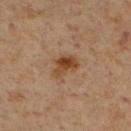biopsy status: total-body-photography surveillance lesion; no biopsy
tile lighting: cross-polarized illumination
patient: male, roughly 60 years of age
automated metrics: an area of roughly 5.5 mm², an outline eccentricity of about 0.8 (0 = round, 1 = elongated), and two-axis asymmetry of about 0.4; a lesion color around L≈41 a*≈19 b*≈32 in CIELAB and roughly 10 lightness units darker than nearby skin
anatomic site: the right lower leg
size: ~3.5 mm (longest diameter)
acquisition: 15 mm crop, total-body photography